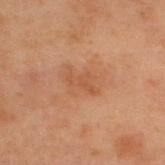Q: Was a biopsy performed?
A: imaged on a skin check; not biopsied
Q: What is the imaging modality?
A: 15 mm crop, total-body photography
Q: Lesion size?
A: ≈3.5 mm
Q: Who is the patient?
A: male, aged approximately 55
Q: Where on the body is the lesion?
A: the back
Q: Illumination type?
A: cross-polarized